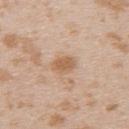| feature | finding |
|---|---|
| follow-up | no biopsy performed (imaged during a skin exam) |
| anatomic site | the upper back |
| subject | female, aged around 25 |
| illumination | white-light |
| image | total-body-photography crop, ~15 mm field of view |